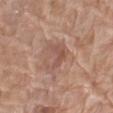Located on the right thigh.
A female patient, aged around 75.
A 15 mm crop from a total-body photograph taken for skin-cancer surveillance.